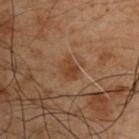| field | value |
|---|---|
| biopsy status | no biopsy performed (imaged during a skin exam) |
| automated metrics | a footprint of about 4.5 mm², an eccentricity of roughly 0.55, and a symmetry-axis asymmetry near 0.25; a mean CIELAB color near L≈31 a*≈16 b*≈26, about 6 CIELAB-L* units darker than the surrounding skin, and a lesion-to-skin contrast of about 6.5 (normalized; higher = more distinct); a classifier nevus-likeness of about 5/100 and a detector confidence of about 100 out of 100 that the crop contains a lesion |
| size | about 2.5 mm |
| imaging modality | total-body-photography crop, ~15 mm field of view |
| patient | male, in their 50s |
| body site | the right upper arm |